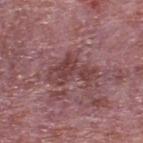Imaged during a routine full-body skin examination; the lesion was not biopsied and no histopathology is available.
Captured under white-light illumination.
A lesion tile, about 15 mm wide, cut from a 3D total-body photograph.
On the back.
A male patient in their mid-70s.
Measured at roughly 5.5 mm in maximum diameter.